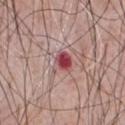workup = catalogued during a skin exam; not biopsied
acquisition = ~15 mm tile from a whole-body skin photo
patient = male, roughly 60 years of age
site = the chest